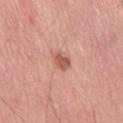{"biopsy_status": "not biopsied; imaged during a skin examination", "patient": {"sex": "male", "age_approx": 70}, "image": {"source": "total-body photography crop", "field_of_view_mm": 15}, "lesion_size": {"long_diameter_mm_approx": 2.5}, "lighting": "white-light", "site": "right thigh"}